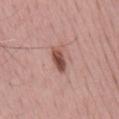Clinical impression: Part of a total-body skin-imaging series; this lesion was reviewed on a skin check and was not flagged for biopsy. Context: The lesion is on the mid back. A 15 mm close-up extracted from a 3D total-body photography capture. This is a white-light tile. The subject is a male aged around 70. Longest diameter approximately 3.5 mm. The total-body-photography lesion software estimated a lesion color around L≈51 a*≈23 b*≈25 in CIELAB, a lesion–skin lightness drop of about 14, and a normalized border contrast of about 9.5. The analysis additionally found internal color variation of about 6 on a 0–10 scale and a peripheral color-asymmetry measure near 2.5. And it measured a nevus-likeness score of about 70/100 and a detector confidence of about 100 out of 100 that the crop contains a lesion.